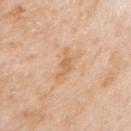biopsy status: total-body-photography surveillance lesion; no biopsy | patient: male, about 75 years old | imaging modality: ~15 mm tile from a whole-body skin photo | size: ~3.5 mm (longest diameter) | lighting: white-light illumination | location: the arm.